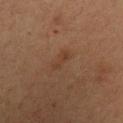Recorded during total-body skin imaging; not selected for excision or biopsy.
The recorded lesion diameter is about 3 mm.
The lesion is on the chest.
A male patient, roughly 35 years of age.
Cropped from a whole-body photographic skin survey; the tile spans about 15 mm.
Captured under cross-polarized illumination.